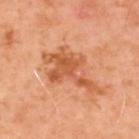| field | value |
|---|---|
| biopsy status | imaged on a skin check; not biopsied |
| diameter | about 7.5 mm |
| body site | the upper back |
| illumination | cross-polarized |
| subject | male, approximately 50 years of age |
| image source | ~15 mm crop, total-body skin-cancer survey |